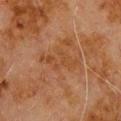Q: Was a biopsy performed?
A: no biopsy performed (imaged during a skin exam)
Q: Who is the patient?
A: male, roughly 80 years of age
Q: What is the anatomic site?
A: the upper back
Q: Illumination type?
A: cross-polarized
Q: How large is the lesion?
A: about 5 mm
Q: What is the imaging modality?
A: ~15 mm tile from a whole-body skin photo
Q: What did automated image analysis measure?
A: a footprint of about 8 mm², a shape eccentricity near 0.9, and a shape-asymmetry score of about 0.45 (0 = symmetric); a lesion color around L≈36 a*≈19 b*≈30 in CIELAB and a lesion–skin lightness drop of about 4; a nevus-likeness score of about 0/100 and lesion-presence confidence of about 100/100